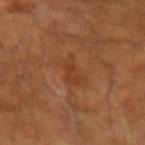No biopsy was performed on this lesion — it was imaged during a full skin examination and was not determined to be concerning. The lesion's longest dimension is about 3.5 mm. Imaged with cross-polarized lighting. A male subject, aged 63–67. A close-up tile cropped from a whole-body skin photograph, about 15 mm across.